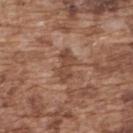No biopsy was performed on this lesion — it was imaged during a full skin examination and was not determined to be concerning.
The subject is a male in their mid- to late 70s.
A 15 mm crop from a total-body photograph taken for skin-cancer surveillance.
The lesion-visualizer software estimated an area of roughly 5.5 mm², an outline eccentricity of about 0.9 (0 = round, 1 = elongated), and a symmetry-axis asymmetry near 0.25. And it measured a lesion color around L≈45 a*≈21 b*≈29 in CIELAB and a normalized lesion–skin contrast near 7. The analysis additionally found a border-irregularity index near 3.5/10. It also reported lesion-presence confidence of about 85/100.
From the upper back.
Approximately 4 mm at its widest.